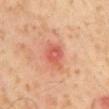biopsy status = total-body-photography surveillance lesion; no biopsy
anatomic site = the mid back
lesion size = ~3.5 mm (longest diameter)
lighting = cross-polarized illumination
imaging modality = total-body-photography crop, ~15 mm field of view
patient = male, in their 60s